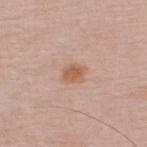Impression: The lesion was photographed on a routine skin check and not biopsied; there is no pathology result. Clinical summary: The tile uses white-light illumination. From the upper back. Approximately 2.5 mm at its widest. Automated tile analysis of the lesion measured a footprint of about 4.5 mm², an outline eccentricity of about 0.65 (0 = round, 1 = elongated), and a symmetry-axis asymmetry near 0.25. The software also gave a mean CIELAB color near L≈59 a*≈20 b*≈31, roughly 9 lightness units darker than nearby skin, and a lesion-to-skin contrast of about 7.5 (normalized; higher = more distinct). The analysis additionally found an automated nevus-likeness rating near 85 out of 100 and a lesion-detection confidence of about 100/100. A male patient, in their 50s. Cropped from a whole-body photographic skin survey; the tile spans about 15 mm.